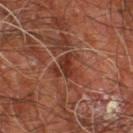Recorded during total-body skin imaging; not selected for excision or biopsy. The recorded lesion diameter is about 3.5 mm. The total-body-photography lesion software estimated about 9 CIELAB-L* units darker than the surrounding skin and a normalized lesion–skin contrast near 8. The software also gave a color-variation rating of about 3.5/10 and radial color variation of about 1. The lesion is located on the right leg. This is a cross-polarized tile. Cropped from a total-body skin-imaging series; the visible field is about 15 mm. A male subject, aged 58 to 62.